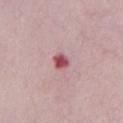{"biopsy_status": "not biopsied; imaged during a skin examination"}